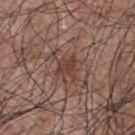  biopsy_status: not biopsied; imaged during a skin examination
  lesion_size:
    long_diameter_mm_approx: 2.5
  image:
    source: total-body photography crop
    field_of_view_mm: 15
  site: upper back
  automated_metrics:
    area_mm2_approx: 4.5
    eccentricity: 0.4
    cielab_L: 40
    cielab_a: 19
    cielab_b: 23
    vs_skin_darker_L: 8.0
  patient:
    sex: male
    age_approx: 70